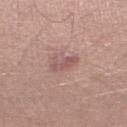Captured during whole-body skin photography for melanoma surveillance; the lesion was not biopsied. Automated image analysis of the tile measured a lesion area of about 5 mm², an eccentricity of roughly 0.9, and a symmetry-axis asymmetry near 0.45. And it measured an average lesion color of about L≈55 a*≈21 b*≈21 (CIELAB), about 9 CIELAB-L* units darker than the surrounding skin, and a lesion-to-skin contrast of about 6 (normalized; higher = more distinct). The analysis additionally found a lesion-detection confidence of about 100/100. A male subject roughly 30 years of age. Cropped from a total-body skin-imaging series; the visible field is about 15 mm. Captured under white-light illumination.